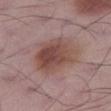Captured during whole-body skin photography for melanoma surveillance; the lesion was not biopsied.